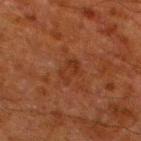biopsy_status: not biopsied; imaged during a skin examination
lesion_size:
  long_diameter_mm_approx: 3.0
site: left lower leg
patient:
  sex: male
  age_approx: 80
image:
  source: total-body photography crop
  field_of_view_mm: 15